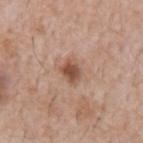Recorded during total-body skin imaging; not selected for excision or biopsy.
Approximately 2.5 mm at its widest.
A male subject aged 58–62.
A region of skin cropped from a whole-body photographic capture, roughly 15 mm wide.
The lesion is located on the chest.
Captured under white-light illumination.